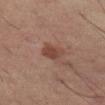{"biopsy_status": "not biopsied; imaged during a skin examination", "lesion_size": {"long_diameter_mm_approx": 3.0}, "site": "left thigh", "patient": {"sex": "male", "age_approx": 60}, "image": {"source": "total-body photography crop", "field_of_view_mm": 15}, "automated_metrics": {"nevus_likeness_0_100": 30}, "lighting": "cross-polarized"}